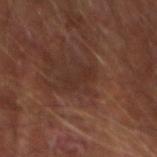Clinical impression: Part of a total-body skin-imaging series; this lesion was reviewed on a skin check and was not flagged for biopsy. Context: Imaged with cross-polarized lighting. On the right forearm. The recorded lesion diameter is about 3.5 mm. The patient is a male aged approximately 65. A 15 mm close-up tile from a total-body photography series done for melanoma screening.